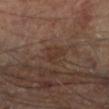Imaged during a routine full-body skin examination; the lesion was not biopsied and no histopathology is available.
From the left lower leg.
Longest diameter approximately 3 mm.
A male subject aged 68–72.
The tile uses cross-polarized illumination.
Cropped from a whole-body photographic skin survey; the tile spans about 15 mm.
Automated image analysis of the tile measured a lesion–skin lightness drop of about 4. The software also gave a classifier nevus-likeness of about 0/100 and a detector confidence of about 100 out of 100 that the crop contains a lesion.